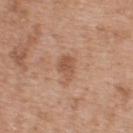  patient:
    sex: male
    age_approx: 55
  lesion_size:
    long_diameter_mm_approx: 2.5
  site: upper back
  image:
    source: total-body photography crop
    field_of_view_mm: 15